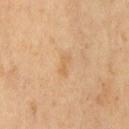{"biopsy_status": "not biopsied; imaged during a skin examination", "patient": {"sex": "male", "age_approx": 65}, "lighting": "cross-polarized", "automated_metrics": {"cielab_L": 62, "cielab_a": 18, "cielab_b": 39, "vs_skin_darker_L": 6.0, "vs_skin_contrast_norm": 4.5, "border_irregularity_0_10": 4.0, "color_variation_0_10": 0.0, "peripheral_color_asymmetry": 0.0, "nevus_likeness_0_100": 0, "lesion_detection_confidence_0_100": 100}, "image": {"source": "total-body photography crop", "field_of_view_mm": 15}}